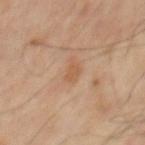Case summary:
– biopsy status: total-body-photography surveillance lesion; no biopsy
– diameter: about 1.5 mm
– location: the mid back
– illumination: cross-polarized illumination
– subject: male, in their mid- to late 50s
– image: 15 mm crop, total-body photography
– TBP lesion metrics: an area of roughly 2 mm², a shape eccentricity near 0.65, and a symmetry-axis asymmetry near 0.3; a lesion color around L≈51 a*≈19 b*≈33 in CIELAB; border irregularity of about 2.5 on a 0–10 scale; a classifier nevus-likeness of about 0/100